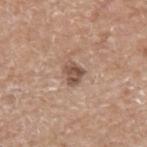  biopsy_status: not biopsied; imaged during a skin examination
  lesion_size:
    long_diameter_mm_approx: 3.0
  site: arm
  lighting: white-light
  image:
    source: total-body photography crop
    field_of_view_mm: 15
  patient:
    sex: male
    age_approx: 65
  automated_metrics:
    cielab_L: 51
    cielab_a: 18
    cielab_b: 26
    vs_skin_contrast_norm: 8.5
    nevus_likeness_0_100: 20
    lesion_detection_confidence_0_100: 100